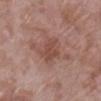<case>
<biopsy_status>not biopsied; imaged during a skin examination</biopsy_status>
<lesion_size>
  <long_diameter_mm_approx>3.5</long_diameter_mm_approx>
</lesion_size>
<lighting>white-light</lighting>
<automated_metrics>
  <border_irregularity_0_10>4.0</border_irregularity_0_10>
  <color_variation_0_10>2.0</color_variation_0_10>
  <peripheral_color_asymmetry>1.0</peripheral_color_asymmetry>
</automated_metrics>
<patient>
  <sex>female</sex>
  <age_approx>70</age_approx>
</patient>
<site>leg</site>
<image>
  <source>total-body photography crop</source>
  <field_of_view_mm>15</field_of_view_mm>
</image>
</case>